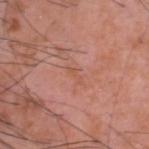notes: catalogued during a skin exam; not biopsied | acquisition: ~15 mm crop, total-body skin-cancer survey | subject: male, roughly 60 years of age | tile lighting: white-light | body site: the head or neck | lesion diameter: ~2.5 mm (longest diameter).